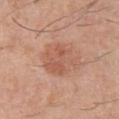Q: Is there a histopathology result?
A: total-body-photography surveillance lesion; no biopsy
Q: Automated lesion metrics?
A: two-axis asymmetry of about 0.2; an average lesion color of about L≈57 a*≈23 b*≈31 (CIELAB), about 8 CIELAB-L* units darker than the surrounding skin, and a lesion-to-skin contrast of about 5.5 (normalized; higher = more distinct)
Q: Patient demographics?
A: male, aged around 30
Q: How large is the lesion?
A: ~5 mm (longest diameter)
Q: How was this image acquired?
A: ~15 mm tile from a whole-body skin photo
Q: Where on the body is the lesion?
A: the chest
Q: Illumination type?
A: white-light